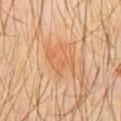{"biopsy_status": "not biopsied; imaged during a skin examination", "patient": {"sex": "male", "age_approx": 30}, "site": "chest", "lighting": "cross-polarized", "automated_metrics": {"cielab_L": 62, "cielab_a": 23, "cielab_b": 39, "vs_skin_darker_L": 5.0, "vs_skin_contrast_norm": 4.0, "nevus_likeness_0_100": 60, "lesion_detection_confidence_0_100": 100}, "image": {"source": "total-body photography crop", "field_of_view_mm": 15}}